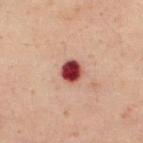| key | value |
|---|---|
| diameter | ≈2.5 mm |
| image | 15 mm crop, total-body photography |
| TBP lesion metrics | an average lesion color of about L≈39 a*≈31 b*≈23 (CIELAB) and roughly 22 lightness units darker than nearby skin; a border-irregularity index near 1/10, a within-lesion color-variation index near 4.5/10, and a peripheral color-asymmetry measure near 1.5; a classifier nevus-likeness of about 0/100 and a detector confidence of about 100 out of 100 that the crop contains a lesion |
| lighting | cross-polarized illumination |
| subject | male, aged around 50 |
| location | the upper back |